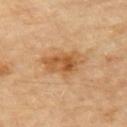Assessment: Imaged during a routine full-body skin examination; the lesion was not biopsied and no histopathology is available. Acquisition and patient details: An algorithmic analysis of the crop reported an average lesion color of about L≈54 a*≈21 b*≈39 (CIELAB), a lesion–skin lightness drop of about 10, and a lesion-to-skin contrast of about 7.5 (normalized; higher = more distinct). It also reported internal color variation of about 5.5 on a 0–10 scale and a peripheral color-asymmetry measure near 2. The software also gave a classifier nevus-likeness of about 55/100 and a detector confidence of about 100 out of 100 that the crop contains a lesion. The lesion's longest dimension is about 5 mm. A roughly 15 mm field-of-view crop from a total-body skin photograph. The lesion is located on the back. Captured under cross-polarized illumination. A male patient aged approximately 85 or older.